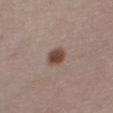The lesion was tiled from a total-body skin photograph and was not biopsied.